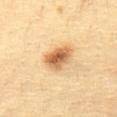This lesion was catalogued during total-body skin photography and was not selected for biopsy.
On the front of the torso.
Measured at roughly 3.5 mm in maximum diameter.
This is a cross-polarized tile.
A female patient roughly 30 years of age.
A region of skin cropped from a whole-body photographic capture, roughly 15 mm wide.